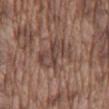Notes:
– workup — total-body-photography surveillance lesion; no biopsy
– automated lesion analysis — an average lesion color of about L≈43 a*≈17 b*≈23 (CIELAB) and a normalized border contrast of about 6.5; a nevus-likeness score of about 0/100 and lesion-presence confidence of about 60/100
– lighting — white-light illumination
– subject — male, roughly 75 years of age
– diameter — ~4 mm (longest diameter)
– location — the mid back
– image source — ~15 mm crop, total-body skin-cancer survey